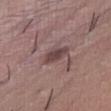The lesion was photographed on a routine skin check and not biopsied; there is no pathology result.
Approximately 3.5 mm at its widest.
The lesion is on the right thigh.
This image is a 15 mm lesion crop taken from a total-body photograph.
The patient is a female aged around 25.
The tile uses white-light illumination.